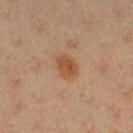Assessment: Captured during whole-body skin photography for melanoma surveillance; the lesion was not biopsied. Acquisition and patient details: About 3 mm across. Imaged with cross-polarized lighting. On the right thigh. A close-up tile cropped from a whole-body skin photograph, about 15 mm across. A female patient, approximately 20 years of age. An algorithmic analysis of the crop reported an area of roughly 6 mm² and a shape eccentricity near 0.6. It also reported a lesion–skin lightness drop of about 8 and a normalized border contrast of about 7.5. The software also gave an automated nevus-likeness rating near 90 out of 100 and a detector confidence of about 100 out of 100 that the crop contains a lesion.